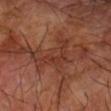follow-up=total-body-photography surveillance lesion; no biopsy | subject=male, in their 70s | anatomic site=the arm | image source=~15 mm tile from a whole-body skin photo.